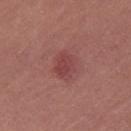No biopsy was performed on this lesion — it was imaged during a full skin examination and was not determined to be concerning. A female patient, aged around 45. About 3.5 mm across. From the leg. The lesion-visualizer software estimated a lesion color around L≈44 a*≈27 b*≈22 in CIELAB, roughly 8 lightness units darker than nearby skin, and a lesion-to-skin contrast of about 6 (normalized; higher = more distinct). It also reported a border-irregularity index near 2/10, a within-lesion color-variation index near 2/10, and peripheral color asymmetry of about 0.5. Cropped from a total-body skin-imaging series; the visible field is about 15 mm. This is a white-light tile.